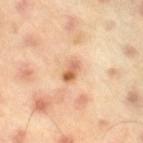biopsy_status: not biopsied; imaged during a skin examination
image:
  source: total-body photography crop
  field_of_view_mm: 15
patient:
  sex: male
  age_approx: 45
site: right thigh